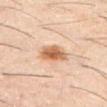follow-up: catalogued during a skin exam; not biopsied | image-analysis metrics: a lesion color around L≈58 a*≈21 b*≈34 in CIELAB, about 13 CIELAB-L* units darker than the surrounding skin, and a normalized border contrast of about 9.5; a lesion-detection confidence of about 100/100 | location: the abdomen | diameter: about 3.5 mm | image: 15 mm crop, total-body photography | patient: male, in their 60s.